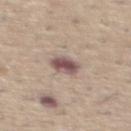Clinical impression: The lesion was tiled from a total-body skin photograph and was not biopsied. Acquisition and patient details: This is a white-light tile. The lesion is located on the abdomen. A 15 mm close-up tile from a total-body photography series done for melanoma screening. A male patient, roughly 60 years of age. Longest diameter approximately 3 mm.